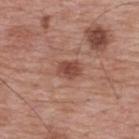<tbp_lesion>
<biopsy_status>not biopsied; imaged during a skin examination</biopsy_status>
<site>upper back</site>
<image>
  <source>total-body photography crop</source>
  <field_of_view_mm>15</field_of_view_mm>
</image>
<lesion_size>
  <long_diameter_mm_approx>2.5</long_diameter_mm_approx>
</lesion_size>
<automated_metrics>
  <area_mm2_approx>4.5</area_mm2_approx>
  <eccentricity>0.65</eccentricity>
  <border_irregularity_0_10>2.0</border_irregularity_0_10>
  <color_variation_0_10>2.5</color_variation_0_10>
  <peripheral_color_asymmetry>0.5</peripheral_color_asymmetry>
</automated_metrics>
<patient>
  <sex>male</sex>
  <age_approx>70</age_approx>
</patient>
<lighting>white-light</lighting>
</tbp_lesion>